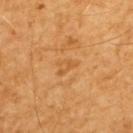Recorded during total-body skin imaging; not selected for excision or biopsy. The subject is a male approximately 60 years of age. From the upper back. A 15 mm close-up extracted from a 3D total-body photography capture.